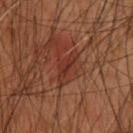Assessment:
This lesion was catalogued during total-body skin photography and was not selected for biopsy.
Context:
Cropped from a total-body skin-imaging series; the visible field is about 15 mm. Located on the upper back. The patient is a male roughly 60 years of age.